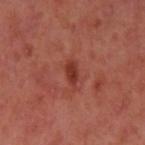Clinical impression: The lesion was tiled from a total-body skin photograph and was not biopsied. Context: Captured under cross-polarized illumination. The lesion-visualizer software estimated an eccentricity of roughly 0.85 and a symmetry-axis asymmetry near 0.25. And it measured a lesion color around L≈36 a*≈31 b*≈29 in CIELAB and a normalized lesion–skin contrast near 8. The analysis additionally found a border-irregularity rating of about 2.5/10, a color-variation rating of about 1.5/10, and radial color variation of about 0.5. The software also gave lesion-presence confidence of about 100/100. Cropped from a total-body skin-imaging series; the visible field is about 15 mm. The patient is a female approximately 40 years of age. On the left upper arm. Approximately 2.5 mm at its widest.